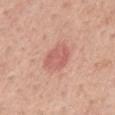Findings:
– image — ~15 mm tile from a whole-body skin photo
– lighting — white-light
– anatomic site — the mid back
– patient — male, aged 53–57
– lesion size — about 3.5 mm